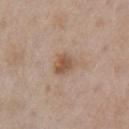Impression: The lesion was tiled from a total-body skin photograph and was not biopsied. Context: About 3 mm across. The patient is a male aged approximately 55. From the right upper arm. A lesion tile, about 15 mm wide, cut from a 3D total-body photograph.